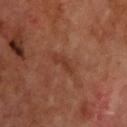The lesion was tiled from a total-body skin photograph and was not biopsied. Imaged with cross-polarized lighting. The lesion is located on the upper back. The lesion's longest dimension is about 3 mm. Automated tile analysis of the lesion measured a lesion area of about 3 mm², a shape eccentricity near 0.9, and a symmetry-axis asymmetry near 0.5. And it measured a lesion color around L≈36 a*≈25 b*≈30 in CIELAB and roughly 6 lightness units darker than nearby skin. The software also gave a border-irregularity rating of about 6/10, a color-variation rating of about 0/10, and a peripheral color-asymmetry measure near 0. The software also gave a nevus-likeness score of about 0/100. A 15 mm close-up extracted from a 3D total-body photography capture. A male subject, about 70 years old.